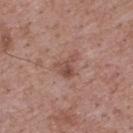Impression: Captured during whole-body skin photography for melanoma surveillance; the lesion was not biopsied. Background: The patient is a male about 70 years old. The lesion is on the upper back. Automated image analysis of the tile measured an average lesion color of about L≈49 a*≈21 b*≈25 (CIELAB) and roughly 9 lightness units darker than nearby skin. The software also gave a border-irregularity index near 5/10, a within-lesion color-variation index near 5/10, and peripheral color asymmetry of about 2. The analysis additionally found a nevus-likeness score of about 5/100 and a lesion-detection confidence of about 100/100. This image is a 15 mm lesion crop taken from a total-body photograph.